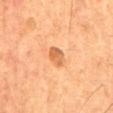Assessment:
No biopsy was performed on this lesion — it was imaged during a full skin examination and was not determined to be concerning.
Acquisition and patient details:
The patient is a male approximately 55 years of age. From the chest. A 15 mm close-up tile from a total-body photography series done for melanoma screening.